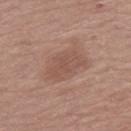Part of a total-body skin-imaging series; this lesion was reviewed on a skin check and was not flagged for biopsy. An algorithmic analysis of the crop reported a mean CIELAB color near L≈52 a*≈19 b*≈26 and a normalized border contrast of about 5. And it measured border irregularity of about 2.5 on a 0–10 scale, internal color variation of about 2 on a 0–10 scale, and radial color variation of about 0.5. Located on the right thigh. About 5 mm across. The patient is a female aged around 65. A 15 mm close-up extracted from a 3D total-body photography capture.